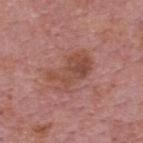A male subject about 75 years old. On the upper back. This image is a 15 mm lesion crop taken from a total-body photograph. Imaged with white-light lighting.